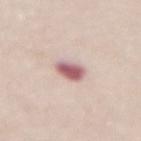Q: Was this lesion biopsied?
A: catalogued during a skin exam; not biopsied
Q: How large is the lesion?
A: about 3 mm
Q: What are the patient's age and sex?
A: female, about 70 years old
Q: What is the anatomic site?
A: the abdomen
Q: What is the imaging modality?
A: ~15 mm crop, total-body skin-cancer survey
Q: Automated lesion metrics?
A: internal color variation of about 5 on a 0–10 scale and radial color variation of about 1.5; an automated nevus-likeness rating near 5 out of 100
Q: How was the tile lit?
A: white-light